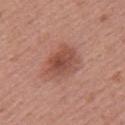Assessment: Captured during whole-body skin photography for melanoma surveillance; the lesion was not biopsied. Background: Imaged with white-light lighting. A lesion tile, about 15 mm wide, cut from a 3D total-body photograph. The lesion-visualizer software estimated an outline eccentricity of about 0.6 (0 = round, 1 = elongated). The software also gave an average lesion color of about L≈50 a*≈24 b*≈27 (CIELAB), roughly 10 lightness units darker than nearby skin, and a lesion-to-skin contrast of about 7 (normalized; higher = more distinct). The analysis additionally found border irregularity of about 2.5 on a 0–10 scale, a within-lesion color-variation index near 4.5/10, and peripheral color asymmetry of about 1. It also reported a classifier nevus-likeness of about 65/100. The recorded lesion diameter is about 4.5 mm. Located on the left upper arm. A female subject about 50 years old.